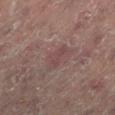{"biopsy_status": "not biopsied; imaged during a skin examination", "image": {"source": "total-body photography crop", "field_of_view_mm": 15}, "patient": {"sex": "female", "age_approx": 80}, "site": "right leg"}